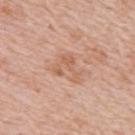The lesion was photographed on a routine skin check and not biopsied; there is no pathology result.